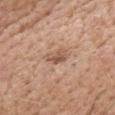Imaged during a routine full-body skin examination; the lesion was not biopsied and no histopathology is available.
The lesion is located on the head or neck.
A male subject in their 60s.
A 15 mm crop from a total-body photograph taken for skin-cancer surveillance.
Captured under white-light illumination.